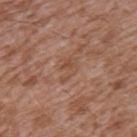No biopsy was performed on this lesion — it was imaged during a full skin examination and was not determined to be concerning. Cropped from a total-body skin-imaging series; the visible field is about 15 mm. The subject is a male aged around 45. Located on the left upper arm.